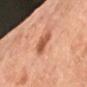Q: Is there a histopathology result?
A: no biopsy performed (imaged during a skin exam)
Q: What is the lesion's diameter?
A: ~3 mm (longest diameter)
Q: Lesion location?
A: the right forearm
Q: How was this image acquired?
A: ~15 mm tile from a whole-body skin photo
Q: Patient demographics?
A: female, aged around 65